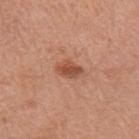<case>
<biopsy_status>not biopsied; imaged during a skin examination</biopsy_status>
<patient>
  <sex>female</sex>
  <age_approx>65</age_approx>
</patient>
<site>left arm</site>
<image>
  <source>total-body photography crop</source>
  <field_of_view_mm>15</field_of_view_mm>
</image>
<lesion_size>
  <long_diameter_mm_approx>3.0</long_diameter_mm_approx>
</lesion_size>
</case>